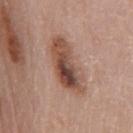<lesion>
<biopsy_status>not biopsied; imaged during a skin examination</biopsy_status>
<lesion_size>
  <long_diameter_mm_approx>7.0</long_diameter_mm_approx>
</lesion_size>
<site>chest</site>
<lighting>white-light</lighting>
<image>
  <source>total-body photography crop</source>
  <field_of_view_mm>15</field_of_view_mm>
</image>
<patient>
  <sex>male</sex>
  <age_approx>80</age_approx>
</patient>
<automated_metrics>
  <cielab_L>49</cielab_L>
  <cielab_a>20</cielab_a>
  <cielab_b>27</cielab_b>
  <vs_skin_darker_L>13.0</vs_skin_darker_L>
  <border_irregularity_0_10>4.0</border_irregularity_0_10>
  <color_variation_0_10>10.0</color_variation_0_10>
  <peripheral_color_asymmetry>3.5</peripheral_color_asymmetry>
  <nevus_likeness_0_100>90</nevus_likeness_0_100>
  <lesion_detection_confidence_0_100>100</lesion_detection_confidence_0_100>
</automated_metrics>
</lesion>